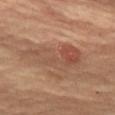Clinical summary: This is a cross-polarized tile. An algorithmic analysis of the crop reported a lesion–skin lightness drop of about 8 and a normalized lesion–skin contrast near 6. It also reported a detector confidence of about 100 out of 100 that the crop contains a lesion. The lesion is on the left thigh. Cropped from a total-body skin-imaging series; the visible field is about 15 mm. The recorded lesion diameter is about 7.5 mm. A female subject, aged 68 to 72.